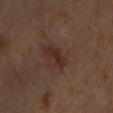Imaged during a routine full-body skin examination; the lesion was not biopsied and no histopathology is available. Automated image analysis of the tile measured a lesion color around L≈26 a*≈18 b*≈23 in CIELAB, roughly 7 lightness units darker than nearby skin, and a lesion-to-skin contrast of about 8.5 (normalized; higher = more distinct). A region of skin cropped from a whole-body photographic capture, roughly 15 mm wide. The lesion is on the chest. The tile uses cross-polarized illumination. A male subject, aged around 45.